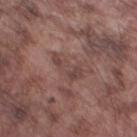follow-up — total-body-photography surveillance lesion; no biopsy
size — about 3.5 mm
subject — male, aged approximately 75
illumination — white-light
TBP lesion metrics — an area of roughly 6 mm² and an outline eccentricity of about 0.8 (0 = round, 1 = elongated); a nevus-likeness score of about 0/100 and lesion-presence confidence of about 95/100
anatomic site — the leg
acquisition — 15 mm crop, total-body photography